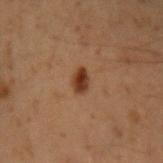biopsy_status: not biopsied; imaged during a skin examination
automated_metrics:
  cielab_L: 33
  cielab_a: 21
  cielab_b: 29
  vs_skin_darker_L: 12.0
  vs_skin_contrast_norm: 10.5
  border_irregularity_0_10: 2.5
  color_variation_0_10: 3.5
  peripheral_color_asymmetry: 1.5
  nevus_likeness_0_100: 100
  lesion_detection_confidence_0_100: 100
lesion_size:
  long_diameter_mm_approx: 2.5
lighting: cross-polarized
image:
  source: total-body photography crop
  field_of_view_mm: 15
site: arm
patient:
  sex: male
  age_approx: 50